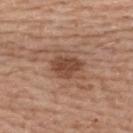Assessment:
Recorded during total-body skin imaging; not selected for excision or biopsy.
Background:
Approximately 3.5 mm at its widest. A region of skin cropped from a whole-body photographic capture, roughly 15 mm wide. A female patient, aged 58–62. From the upper back. Imaged with white-light lighting.